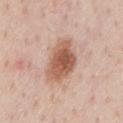notes: catalogued during a skin exam; not biopsied
patient: male, aged 58 to 62
size: ≈6 mm
location: the chest
imaging modality: total-body-photography crop, ~15 mm field of view
TBP lesion metrics: a lesion area of about 16 mm², an outline eccentricity of about 0.8 (0 = round, 1 = elongated), and two-axis asymmetry of about 0.25; a border-irregularity index near 2.5/10 and internal color variation of about 5 on a 0–10 scale; a nevus-likeness score of about 95/100